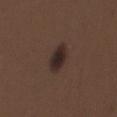Imaged during a routine full-body skin examination; the lesion was not biopsied and no histopathology is available. From the upper back. A 15 mm close-up tile from a total-body photography series done for melanoma screening. The recorded lesion diameter is about 4 mm. A male patient, aged approximately 30. This is a white-light tile.